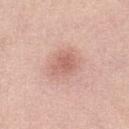Recorded during total-body skin imaging; not selected for excision or biopsy.
The lesion-visualizer software estimated an average lesion color of about L≈61 a*≈24 b*≈27 (CIELAB), about 9 CIELAB-L* units darker than the surrounding skin, and a lesion-to-skin contrast of about 6 (normalized; higher = more distinct). The analysis additionally found border irregularity of about 2.5 on a 0–10 scale, a color-variation rating of about 2.5/10, and radial color variation of about 1. And it measured lesion-presence confidence of about 100/100.
From the abdomen.
A 15 mm close-up extracted from a 3D total-body photography capture.
Approximately 3 mm at its widest.
This is a white-light tile.
A female patient in their mid-60s.